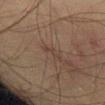<case>
<biopsy_status>not biopsied; imaged during a skin examination</biopsy_status>
<automated_metrics>
  <area_mm2_approx>3.0</area_mm2_approx>
  <eccentricity>0.9</eccentricity>
  <shape_asymmetry>0.5</shape_asymmetry>
  <border_irregularity_0_10>5.5</border_irregularity_0_10>
  <color_variation_0_10>0.0</color_variation_0_10>
  <nevus_likeness_0_100>0</nevus_likeness_0_100>
  <lesion_detection_confidence_0_100>95</lesion_detection_confidence_0_100>
</automated_metrics>
<lighting>cross-polarized</lighting>
<image>
  <source>total-body photography crop</source>
  <field_of_view_mm>15</field_of_view_mm>
</image>
<patient>
  <sex>male</sex>
  <age_approx>40</age_approx>
</patient>
<lesion_size>
  <long_diameter_mm_approx>3.0</long_diameter_mm_approx>
</lesion_size>
<site>left thigh</site>
</case>